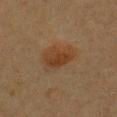Assessment: No biopsy was performed on this lesion — it was imaged during a full skin examination and was not determined to be concerning. Background: Automated tile analysis of the lesion measured a lesion area of about 7.5 mm², an outline eccentricity of about 0.8 (0 = round, 1 = elongated), and a shape-asymmetry score of about 0.25 (0 = symmetric). And it measured about 7 CIELAB-L* units darker than the surrounding skin and a normalized lesion–skin contrast near 7.5. The analysis additionally found a lesion-detection confidence of about 100/100. The subject is a female about 40 years old. A region of skin cropped from a whole-body photographic capture, roughly 15 mm wide. The lesion is located on the chest. Captured under cross-polarized illumination. About 4 mm across.